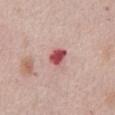<lesion>
<biopsy_status>not biopsied; imaged during a skin examination</biopsy_status>
<patient>
  <sex>male</sex>
  <age_approx>65</age_approx>
</patient>
<lesion_size>
  <long_diameter_mm_approx>2.5</long_diameter_mm_approx>
</lesion_size>
<automated_metrics>
  <area_mm2_approx>4.5</area_mm2_approx>
  <eccentricity>0.7</eccentricity>
  <shape_asymmetry>0.3</shape_asymmetry>
  <cielab_L>52</cielab_L>
  <cielab_a>33</cielab_a>
  <cielab_b>23</cielab_b>
  <vs_skin_contrast_norm>11.0</vs_skin_contrast_norm>
  <lesion_detection_confidence_0_100>100</lesion_detection_confidence_0_100>
</automated_metrics>
<site>abdomen</site>
<image>
  <source>total-body photography crop</source>
  <field_of_view_mm>15</field_of_view_mm>
</image>
</lesion>